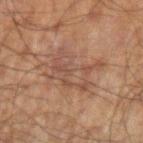Impression: Captured during whole-body skin photography for melanoma surveillance; the lesion was not biopsied. Image and clinical context: The lesion is on the left upper arm. About 5.5 mm across. A 15 mm crop from a total-body photograph taken for skin-cancer surveillance. A male patient, aged 68 to 72.